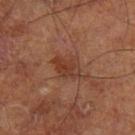notes: total-body-photography surveillance lesion; no biopsy | imaging modality: ~15 mm tile from a whole-body skin photo | location: the leg | lighting: cross-polarized illumination | diameter: ~3.5 mm (longest diameter) | patient: male, aged 68–72 | TBP lesion metrics: a lesion color around L≈36 a*≈22 b*≈28 in CIELAB, about 8 CIELAB-L* units darker than the surrounding skin, and a lesion-to-skin contrast of about 7 (normalized; higher = more distinct); a border-irregularity rating of about 2.5/10, internal color variation of about 3.5 on a 0–10 scale, and radial color variation of about 1; an automated nevus-likeness rating near 10 out of 100 and a detector confidence of about 100 out of 100 that the crop contains a lesion.